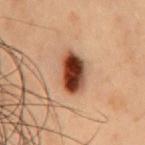Q: What are the patient's age and sex?
A: male, aged 53–57
Q: What did automated image analysis measure?
A: a border-irregularity index near 1.5/10; a classifier nevus-likeness of about 100/100 and a lesion-detection confidence of about 100/100
Q: Illumination type?
A: cross-polarized
Q: Lesion size?
A: ≈5 mm
Q: How was this image acquired?
A: 15 mm crop, total-body photography
Q: Where on the body is the lesion?
A: the chest